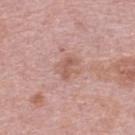Assessment: No biopsy was performed on this lesion — it was imaged during a full skin examination and was not determined to be concerning. Image and clinical context: The lesion is on the upper back. A female patient, aged 63–67. A roughly 15 mm field-of-view crop from a total-body skin photograph. The recorded lesion diameter is about 3 mm. Captured under white-light illumination.